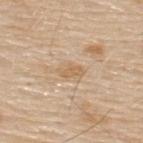Q: Is there a histopathology result?
A: total-body-photography surveillance lesion; no biopsy
Q: Lesion size?
A: about 3 mm
Q: Lesion location?
A: the upper back
Q: How was this image acquired?
A: ~15 mm tile from a whole-body skin photo
Q: How was the tile lit?
A: white-light
Q: Patient demographics?
A: male, aged approximately 80